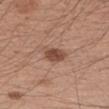The lesion was tiled from a total-body skin photograph and was not biopsied. The lesion's longest dimension is about 3 mm. Captured under white-light illumination. A male subject aged around 60. A 15 mm close-up tile from a total-body photography series done for melanoma screening. Automated tile analysis of the lesion measured an average lesion color of about L≈48 a*≈21 b*≈28 (CIELAB) and roughly 12 lightness units darker than nearby skin. It also reported border irregularity of about 3 on a 0–10 scale, a color-variation rating of about 2.5/10, and radial color variation of about 0.5. On the arm.